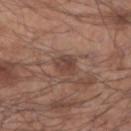{
  "biopsy_status": "not biopsied; imaged during a skin examination",
  "patient": {
    "sex": "male",
    "age_approx": 55
  },
  "image": {
    "source": "total-body photography crop",
    "field_of_view_mm": 15
  },
  "lesion_size": {
    "long_diameter_mm_approx": 3.5
  },
  "site": "right forearm"
}